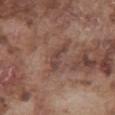Imaged during a routine full-body skin examination; the lesion was not biopsied and no histopathology is available.
Imaged with white-light lighting.
Longest diameter approximately 4 mm.
A close-up tile cropped from a whole-body skin photograph, about 15 mm across.
Located on the abdomen.
A male patient, approximately 75 years of age.